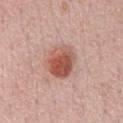{
  "biopsy_status": "not biopsied; imaged during a skin examination",
  "image": {
    "source": "total-body photography crop",
    "field_of_view_mm": 15
  },
  "automated_metrics": {
    "vs_skin_darker_L": 13.0,
    "vs_skin_contrast_norm": 9.0,
    "nevus_likeness_0_100": 100
  },
  "lesion_size": {
    "long_diameter_mm_approx": 4.5
  },
  "patient": {
    "sex": "male",
    "age_approx": 60
  },
  "lighting": "white-light",
  "site": "chest"
}